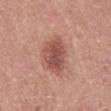notes — catalogued during a skin exam; not biopsied | diameter — ~6 mm (longest diameter) | site — the mid back | patient — male, aged 48–52 | imaging modality — ~15 mm crop, total-body skin-cancer survey | tile lighting — white-light illumination.